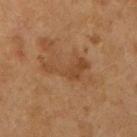– follow-up — catalogued during a skin exam; not biopsied
– illumination — cross-polarized
– body site — the right upper arm
– acquisition — ~15 mm crop, total-body skin-cancer survey
– lesion size — ~5.5 mm (longest diameter)
– subject — female, aged 58–62
– image-analysis metrics — an eccentricity of roughly 0.9; a lesion color around L≈39 a*≈18 b*≈31 in CIELAB and roughly 7 lightness units darker than nearby skin